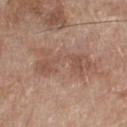{
  "biopsy_status": "not biopsied; imaged during a skin examination",
  "site": "chest",
  "patient": {
    "sex": "male",
    "age_approx": 80
  },
  "automated_metrics": {
    "shape_asymmetry": 0.45
  },
  "image": {
    "source": "total-body photography crop",
    "field_of_view_mm": 15
  },
  "lighting": "white-light"
}